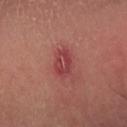lighting: cross-polarized
location: the arm
imaging modality: 15 mm crop, total-body photography
subject: male, in their 40s
lesion diameter: ~3 mm (longest diameter)